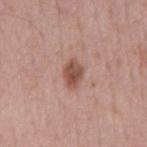Impression:
Captured during whole-body skin photography for melanoma surveillance; the lesion was not biopsied.
Acquisition and patient details:
The subject is a male aged approximately 55. Approximately 3.5 mm at its widest. A close-up tile cropped from a whole-body skin photograph, about 15 mm across. Located on the mid back. Imaged with white-light lighting.